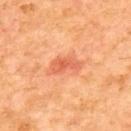<tbp_lesion>
  <biopsy_status>not biopsied; imaged during a skin examination</biopsy_status>
  <site>upper back</site>
  <lesion_size>
    <long_diameter_mm_approx>3.5</long_diameter_mm_approx>
  </lesion_size>
  <image>
    <source>total-body photography crop</source>
    <field_of_view_mm>15</field_of_view_mm>
  </image>
  <patient>
    <sex>male</sex>
    <age_approx>55</age_approx>
  </patient>
  <lighting>cross-polarized</lighting>
  <automated_metrics>
    <border_irregularity_0_10>3.5</border_irregularity_0_10>
    <color_variation_0_10>3.0</color_variation_0_10>
    <peripheral_color_asymmetry>1.0</peripheral_color_asymmetry>
  </automated_metrics>
</tbp_lesion>